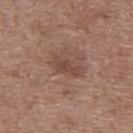Q: Is there a histopathology result?
A: total-body-photography surveillance lesion; no biopsy
Q: How large is the lesion?
A: ≈4 mm
Q: Automated lesion metrics?
A: a footprint of about 5 mm², a shape eccentricity near 0.9, and a symmetry-axis asymmetry near 0.35; about 8 CIELAB-L* units darker than the surrounding skin
Q: Where on the body is the lesion?
A: the upper back
Q: What kind of image is this?
A: 15 mm crop, total-body photography
Q: How was the tile lit?
A: white-light
Q: Who is the patient?
A: male, roughly 60 years of age